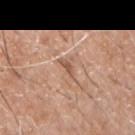| feature | finding |
|---|---|
| biopsy status | total-body-photography surveillance lesion; no biopsy |
| subject | male, about 40 years old |
| lighting | white-light |
| image source | ~15 mm tile from a whole-body skin photo |
| size | ≈2.5 mm |
| site | the chest |